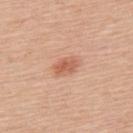The lesion was tiled from a total-body skin photograph and was not biopsied. A male subject, aged 58–62. This is a white-light tile. The lesion is located on the upper back. Approximately 3.5 mm at its widest. A 15 mm close-up tile from a total-body photography series done for melanoma screening.